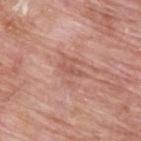Q: Was a biopsy performed?
A: no biopsy performed (imaged during a skin exam)
Q: Lesion location?
A: the upper back
Q: Lesion size?
A: about 2.5 mm
Q: What are the patient's age and sex?
A: male, aged 63–67
Q: What kind of image is this?
A: 15 mm crop, total-body photography